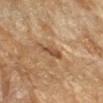Case summary:
• workup · imaged on a skin check; not biopsied
• acquisition · total-body-photography crop, ~15 mm field of view
• patient · female, about 70 years old
• anatomic site · the right forearm